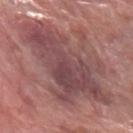Q: Was a biopsy performed?
A: catalogued during a skin exam; not biopsied
Q: Who is the patient?
A: female, aged around 60
Q: Lesion location?
A: the left forearm
Q: What did automated image analysis measure?
A: an average lesion color of about L≈46 a*≈22 b*≈18 (CIELAB) and a normalized lesion–skin contrast near 8; a nevus-likeness score of about 0/100 and a lesion-detection confidence of about 70/100
Q: What lighting was used for the tile?
A: white-light illumination
Q: What kind of image is this?
A: ~15 mm tile from a whole-body skin photo